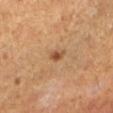No biopsy was performed on this lesion — it was imaged during a full skin examination and was not determined to be concerning. This image is a 15 mm lesion crop taken from a total-body photograph. The tile uses cross-polarized illumination. Automated image analysis of the tile measured border irregularity of about 2 on a 0–10 scale, a within-lesion color-variation index near 1.5/10, and peripheral color asymmetry of about 0.5. Longest diameter approximately 1.5 mm. On the leg. The patient is a male in their mid- to late 60s.